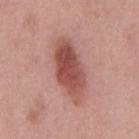TBP lesion metrics: an area of roughly 17 mm², a shape eccentricity near 0.9, and a symmetry-axis asymmetry near 0.15; a lesion color around L≈51 a*≈27 b*≈25 in CIELAB, roughly 14 lightness units darker than nearby skin, and a lesion-to-skin contrast of about 9.5 (normalized; higher = more distinct); a border-irregularity rating of about 2.5/10, a within-lesion color-variation index near 5.5/10, and peripheral color asymmetry of about 2
site: the mid back
lesion diameter: ~7 mm (longest diameter)
subject: male, approximately 40 years of age
tile lighting: white-light
acquisition: total-body-photography crop, ~15 mm field of view